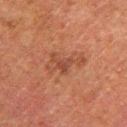workup=total-body-photography surveillance lesion; no biopsy
image=~15 mm crop, total-body skin-cancer survey
tile lighting=cross-polarized
body site=the leg
patient=male, aged around 80
lesion diameter=about 5 mm